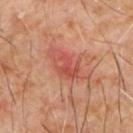Recorded during total-body skin imaging; not selected for excision or biopsy. A 15 mm crop from a total-body photograph taken for skin-cancer surveillance. The total-body-photography lesion software estimated an area of roughly 7 mm², a shape eccentricity near 0.8, and two-axis asymmetry of about 0.3. The lesion's longest dimension is about 4 mm. This is a cross-polarized tile. A male patient, roughly 60 years of age. On the chest.